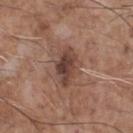biopsy_status: not biopsied; imaged during a skin examination
lesion_size:
  long_diameter_mm_approx: 5.0
site: chest
patient:
  sex: male
  age_approx: 70
image:
  source: total-body photography crop
  field_of_view_mm: 15
lighting: white-light